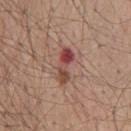Assessment: No biopsy was performed on this lesion — it was imaged during a full skin examination and was not determined to be concerning. Background: The lesion is located on the mid back. About 4.5 mm across. The lesion-visualizer software estimated a lesion area of about 6.5 mm², an outline eccentricity of about 0.95 (0 = round, 1 = elongated), and a symmetry-axis asymmetry near 0.25. It also reported a lesion color around L≈46 a*≈25 b*≈25 in CIELAB, about 12 CIELAB-L* units darker than the surrounding skin, and a lesion-to-skin contrast of about 9 (normalized; higher = more distinct). And it measured a classifier nevus-likeness of about 0/100 and a lesion-detection confidence of about 100/100. A male subject, aged approximately 60. A 15 mm close-up extracted from a 3D total-body photography capture. This is a white-light tile.